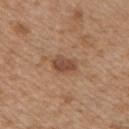Q: Was this lesion biopsied?
A: imaged on a skin check; not biopsied
Q: What are the patient's age and sex?
A: male, aged 48–52
Q: What kind of image is this?
A: ~15 mm crop, total-body skin-cancer survey
Q: What did automated image analysis measure?
A: an area of roughly 5 mm², a shape eccentricity near 0.75, and a shape-asymmetry score of about 0.25 (0 = symmetric); a lesion–skin lightness drop of about 10; radial color variation of about 1; a classifier nevus-likeness of about 85/100
Q: Illumination type?
A: white-light
Q: Where on the body is the lesion?
A: the front of the torso